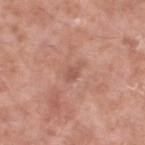| key | value |
|---|---|
| acquisition | ~15 mm tile from a whole-body skin photo |
| tile lighting | white-light |
| TBP lesion metrics | an eccentricity of roughly 0.75 and two-axis asymmetry of about 0.3; a lesion color around L≈55 a*≈23 b*≈27 in CIELAB, about 7 CIELAB-L* units darker than the surrounding skin, and a normalized border contrast of about 5; a classifier nevus-likeness of about 0/100 and lesion-presence confidence of about 100/100 |
| anatomic site | the left lower leg |
| patient | male, aged 53 to 57 |